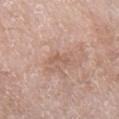Q: Is there a histopathology result?
A: imaged on a skin check; not biopsied
Q: How was this image acquired?
A: total-body-photography crop, ~15 mm field of view
Q: What is the anatomic site?
A: the right lower leg
Q: What are the patient's age and sex?
A: female, approximately 75 years of age